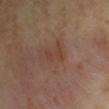Assessment:
The lesion was photographed on a routine skin check and not biopsied; there is no pathology result.
Background:
A lesion tile, about 15 mm wide, cut from a 3D total-body photograph. About 3 mm across. The lesion is on the left upper arm. A male subject, in their mid-40s.